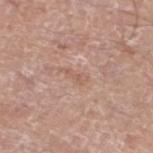biopsy_status: not biopsied; imaged during a skin examination
lighting: white-light
image:
  source: total-body photography crop
  field_of_view_mm: 15
automated_metrics:
  area_mm2_approx: 2.5
  eccentricity: 0.95
  cielab_L: 58
  cielab_a: 20
  cielab_b: 28
  vs_skin_darker_L: 6.0
  vs_skin_contrast_norm: 5.0
  border_irregularity_0_10: 5.0
  color_variation_0_10: 0.0
  peripheral_color_asymmetry: 0.0
patient:
  sex: male
  age_approx: 75
lesion_size:
  long_diameter_mm_approx: 2.5
site: left lower leg